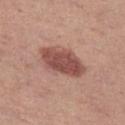Impression: This lesion was catalogued during total-body skin photography and was not selected for biopsy. Image and clinical context: An algorithmic analysis of the crop reported a footprint of about 15 mm², a shape eccentricity near 0.8, and a shape-asymmetry score of about 0.15 (0 = symmetric). It also reported roughly 13 lightness units darker than nearby skin and a lesion-to-skin contrast of about 9 (normalized; higher = more distinct). It also reported a nevus-likeness score of about 75/100 and a lesion-detection confidence of about 100/100. Imaged with white-light lighting. On the leg. A lesion tile, about 15 mm wide, cut from a 3D total-body photograph. Longest diameter approximately 6 mm. A female subject, roughly 40 years of age.